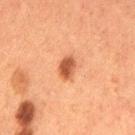biopsy status: total-body-photography surveillance lesion; no biopsy | body site: the left thigh | image-analysis metrics: an area of roughly 4.5 mm² and an outline eccentricity of about 0.75 (0 = round, 1 = elongated); a border-irregularity index near 1.5/10 and a color-variation rating of about 2.5/10; a detector confidence of about 100 out of 100 that the crop contains a lesion | illumination: cross-polarized illumination | diameter: ~3 mm (longest diameter) | imaging modality: 15 mm crop, total-body photography | patient: female, roughly 50 years of age.